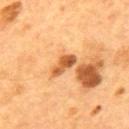Clinical impression:
The lesion was photographed on a routine skin check and not biopsied; there is no pathology result.
Background:
Approximately 3.5 mm at its widest. An algorithmic analysis of the crop reported an average lesion color of about L≈54 a*≈26 b*≈42 (CIELAB). The software also gave border irregularity of about 3.5 on a 0–10 scale, a color-variation rating of about 5/10, and a peripheral color-asymmetry measure near 1.5. The analysis additionally found a nevus-likeness score of about 0/100 and a lesion-detection confidence of about 100/100. Captured under cross-polarized illumination. A close-up tile cropped from a whole-body skin photograph, about 15 mm across. The patient is a male aged approximately 55. The lesion is on the mid back.